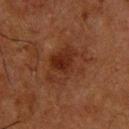<tbp_lesion>
  <biopsy_status>not biopsied; imaged during a skin examination</biopsy_status>
  <image>
    <source>total-body photography crop</source>
    <field_of_view_mm>15</field_of_view_mm>
  </image>
  <site>upper back</site>
  <patient>
    <sex>male</sex>
    <age_approx>65</age_approx>
  </patient>
  <lighting>cross-polarized</lighting>
</tbp_lesion>